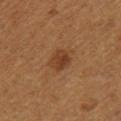The lesion was photographed on a routine skin check and not biopsied; there is no pathology result. The lesion's longest dimension is about 3 mm. From the right thigh. Imaged with cross-polarized lighting. A male patient aged 53 to 57. The total-body-photography lesion software estimated roughly 8 lightness units darker than nearby skin and a normalized border contrast of about 7.5. The software also gave a border-irregularity rating of about 1.5/10, a color-variation rating of about 2.5/10, and a peripheral color-asymmetry measure near 1. The software also gave an automated nevus-likeness rating near 80 out of 100. A 15 mm close-up extracted from a 3D total-body photography capture.